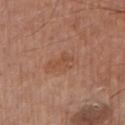The lesion was photographed on a routine skin check and not biopsied; there is no pathology result. Cropped from a total-body skin-imaging series; the visible field is about 15 mm. The recorded lesion diameter is about 3 mm. A male subject, about 65 years old. The lesion is on the chest. Imaged with white-light lighting.